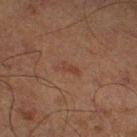{
  "biopsy_status": "not biopsied; imaged during a skin examination",
  "lighting": "cross-polarized",
  "image": {
    "source": "total-body photography crop",
    "field_of_view_mm": 15
  },
  "site": "right thigh",
  "automated_metrics": {
    "cielab_L": 33,
    "cielab_a": 17,
    "cielab_b": 24,
    "vs_skin_darker_L": 4.0,
    "vs_skin_contrast_norm": 4.5
  },
  "patient": {
    "sex": "male",
    "age_approx": 70
  },
  "lesion_size": {
    "long_diameter_mm_approx": 3.0
  }
}